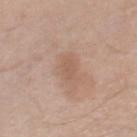Q: Is there a histopathology result?
A: imaged on a skin check; not biopsied
Q: What is the lesion's diameter?
A: ≈3.5 mm
Q: Patient demographics?
A: male, in their mid- to late 60s
Q: What did automated image analysis measure?
A: a lesion area of about 4.5 mm²; an average lesion color of about L≈57 a*≈18 b*≈29 (CIELAB), about 7 CIELAB-L* units darker than the surrounding skin, and a lesion-to-skin contrast of about 5 (normalized; higher = more distinct); a classifier nevus-likeness of about 0/100 and lesion-presence confidence of about 100/100
Q: Lesion location?
A: the right thigh
Q: What kind of image is this?
A: ~15 mm tile from a whole-body skin photo
Q: What lighting was used for the tile?
A: white-light